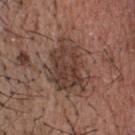This lesion was catalogued during total-body skin photography and was not selected for biopsy.
The patient is a male aged 68 to 72.
Cropped from a total-body skin-imaging series; the visible field is about 15 mm.
Automated tile analysis of the lesion measured a normalized border contrast of about 7.5. It also reported a within-lesion color-variation index near 5/10 and a peripheral color-asymmetry measure near 2.
The lesion is located on the head or neck.
The tile uses white-light illumination.
The lesion's longest dimension is about 7 mm.